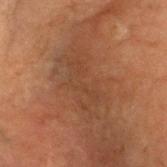<tbp_lesion>
<biopsy_status>not biopsied; imaged during a skin examination</biopsy_status>
<lighting>cross-polarized</lighting>
<patient>
  <sex>male</sex>
  <age_approx>70</age_approx>
</patient>
<image>
  <source>total-body photography crop</source>
  <field_of_view_mm>15</field_of_view_mm>
</image>
<site>head or neck</site>
</tbp_lesion>